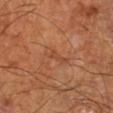automated metrics — an outline eccentricity of about 0.9 (0 = round, 1 = elongated) and a shape-asymmetry score of about 0.35 (0 = symmetric); about 6 CIELAB-L* units darker than the surrounding skin and a lesion-to-skin contrast of about 4.5 (normalized; higher = more distinct); a nevus-likeness score of about 0/100 and a detector confidence of about 100 out of 100 that the crop contains a lesion | size — about 3 mm | site — the right lower leg | illumination — cross-polarized | subject — aged 63 to 67 | image source — ~15 mm tile from a whole-body skin photo.